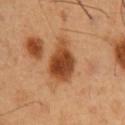Assessment: Recorded during total-body skin imaging; not selected for excision or biopsy. Image and clinical context: On the front of the torso. This image is a 15 mm lesion crop taken from a total-body photograph. The subject is a male aged around 50. An algorithmic analysis of the crop reported an average lesion color of about L≈46 a*≈25 b*≈38 (CIELAB), a lesion–skin lightness drop of about 15, and a lesion-to-skin contrast of about 11 (normalized; higher = more distinct).